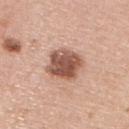Recorded during total-body skin imaging; not selected for excision or biopsy.
The lesion is on the right upper arm.
Imaged with white-light lighting.
This image is a 15 mm lesion crop taken from a total-body photograph.
A female subject approximately 60 years of age.
Approximately 4.5 mm at its widest.